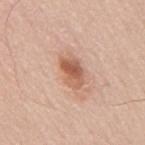From the mid back. A 15 mm close-up tile from a total-body photography series done for melanoma screening. The patient is a male about 60 years old.